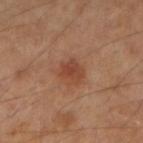No biopsy was performed on this lesion — it was imaged during a full skin examination and was not determined to be concerning.
The tile uses cross-polarized illumination.
About 3 mm across.
Cropped from a total-body skin-imaging series; the visible field is about 15 mm.
The total-body-photography lesion software estimated an area of roughly 5.5 mm², an eccentricity of roughly 0.55, and a symmetry-axis asymmetry near 0.2. The software also gave a border-irregularity index near 2.5/10, a color-variation rating of about 3/10, and radial color variation of about 1. And it measured a nevus-likeness score of about 60/100 and lesion-presence confidence of about 100/100.
The patient is a female aged approximately 60.
Located on the arm.